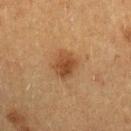Part of a total-body skin-imaging series; this lesion was reviewed on a skin check and was not flagged for biopsy. The lesion's longest dimension is about 3 mm. Cropped from a whole-body photographic skin survey; the tile spans about 15 mm. This is a cross-polarized tile. From the left forearm. A female patient in their 40s.